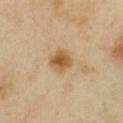<case>
<biopsy_status>not biopsied; imaged during a skin examination</biopsy_status>
<image>
  <source>total-body photography crop</source>
  <field_of_view_mm>15</field_of_view_mm>
</image>
<lesion_size>
  <long_diameter_mm_approx>3.0</long_diameter_mm_approx>
</lesion_size>
<patient>
  <sex>female</sex>
  <age_approx>40</age_approx>
</patient>
<lighting>cross-polarized</lighting>
<site>front of the torso</site>
</case>